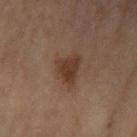Clinical impression:
No biopsy was performed on this lesion — it was imaged during a full skin examination and was not determined to be concerning.
Clinical summary:
This is a cross-polarized tile. The lesion is on the right upper arm. The subject is a female in their 60s. Measured at roughly 4 mm in maximum diameter. A region of skin cropped from a whole-body photographic capture, roughly 15 mm wide. The lesion-visualizer software estimated a footprint of about 8 mm², an outline eccentricity of about 0.6 (0 = round, 1 = elongated), and a symmetry-axis asymmetry near 0.35. The analysis additionally found a border-irregularity rating of about 3.5/10, a color-variation rating of about 2/10, and peripheral color asymmetry of about 0.5. It also reported a classifier nevus-likeness of about 70/100 and a lesion-detection confidence of about 100/100.